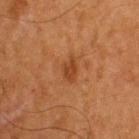Captured during whole-body skin photography for melanoma surveillance; the lesion was not biopsied.
A close-up tile cropped from a whole-body skin photograph, about 15 mm across.
The lesion is on the upper back.
About 3 mm across.
The patient is a male aged 63 to 67.
This is a cross-polarized tile.